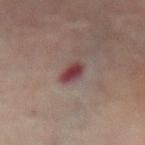Clinical impression:
Part of a total-body skin-imaging series; this lesion was reviewed on a skin check and was not flagged for biopsy.
Background:
On the abdomen. Captured under cross-polarized illumination. A female subject aged around 80. A 15 mm crop from a total-body photograph taken for skin-cancer surveillance.